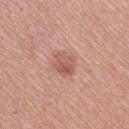Assessment: Recorded during total-body skin imaging; not selected for excision or biopsy. Background: A female patient aged 48 to 52. This is a white-light tile. An algorithmic analysis of the crop reported an automated nevus-likeness rating near 50 out of 100 and lesion-presence confidence of about 100/100. Longest diameter approximately 3 mm. The lesion is on the left thigh. A roughly 15 mm field-of-view crop from a total-body skin photograph.